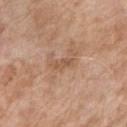| feature | finding |
|---|---|
| biopsy status | no biopsy performed (imaged during a skin exam) |
| lesion size | ≈2.5 mm |
| automated metrics | an eccentricity of roughly 0.9; a lesion color around L≈54 a*≈20 b*≈31 in CIELAB, about 8 CIELAB-L* units darker than the surrounding skin, and a lesion-to-skin contrast of about 5.5 (normalized; higher = more distinct) |
| illumination | white-light illumination |
| location | the right upper arm |
| acquisition | ~15 mm tile from a whole-body skin photo |
| subject | female, aged 73–77 |